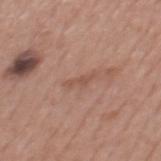body site: the back | automated metrics: a border-irregularity rating of about 5/10 and a color-variation rating of about 0/10; a lesion-detection confidence of about 95/100 | illumination: white-light | image source: total-body-photography crop, ~15 mm field of view | diameter: ~2.5 mm (longest diameter) | patient: male, aged around 55.